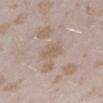This is a white-light tile. The lesion is on the left lower leg. A 15 mm crop from a total-body photograph taken for skin-cancer surveillance. The patient is a female aged 23 to 27.